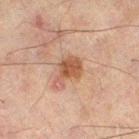{"biopsy_status": "not biopsied; imaged during a skin examination", "site": "left leg", "automated_metrics": {"area_mm2_approx": 7.0, "eccentricity": 0.7, "shape_asymmetry": 0.35, "cielab_L": 41, "cielab_a": 18, "cielab_b": 26}, "lighting": "cross-polarized", "image": {"source": "total-body photography crop", "field_of_view_mm": 15}, "patient": {"sex": "male", "age_approx": 60}}